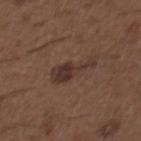<case>
<image>
  <source>total-body photography crop</source>
  <field_of_view_mm>15</field_of_view_mm>
</image>
<site>back</site>
<lesion_size>
  <long_diameter_mm_approx>5.5</long_diameter_mm_approx>
</lesion_size>
<patient>
  <sex>male</sex>
  <age_approx>50</age_approx>
</patient>
</case>